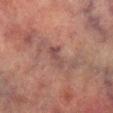This lesion was catalogued during total-body skin photography and was not selected for biopsy. From the right lower leg. Captured under cross-polarized illumination. A male subject aged 73–77. The lesion's longest dimension is about 3 mm. A 15 mm crop from a total-body photograph taken for skin-cancer surveillance. The lesion-visualizer software estimated an area of roughly 4 mm², a shape eccentricity near 0.9, and a shape-asymmetry score of about 0.35 (0 = symmetric). The analysis additionally found a classifier nevus-likeness of about 0/100 and a detector confidence of about 55 out of 100 that the crop contains a lesion.